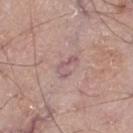Recorded during total-body skin imaging; not selected for excision or biopsy.